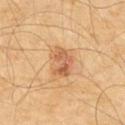Findings:
– notes: total-body-photography surveillance lesion; no biopsy
– automated metrics: an eccentricity of roughly 0.75 and two-axis asymmetry of about 0.25; a mean CIELAB color near L≈57 a*≈23 b*≈37, about 10 CIELAB-L* units darker than the surrounding skin, and a normalized lesion–skin contrast near 7; a border-irregularity index near 3/10 and a peripheral color-asymmetry measure near 2; a classifier nevus-likeness of about 80/100
– subject: male, in their 60s
– lesion diameter: ~3.5 mm (longest diameter)
– site: the chest
– lighting: cross-polarized
– imaging modality: ~15 mm tile from a whole-body skin photo